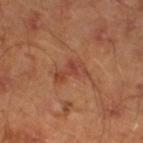Q: Is there a histopathology result?
A: total-body-photography surveillance lesion; no biopsy
Q: What is the anatomic site?
A: the left lower leg
Q: What kind of image is this?
A: ~15 mm tile from a whole-body skin photo
Q: What lighting was used for the tile?
A: cross-polarized
Q: Who is the patient?
A: male, about 45 years old
Q: What is the lesion's diameter?
A: ≈4 mm